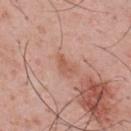image: ~15 mm tile from a whole-body skin photo
patient: male, in their mid-50s
body site: the upper back